A lesion tile, about 15 mm wide, cut from a 3D total-body photograph. A male subject, aged 53 to 57. The lesion is located on the upper back — 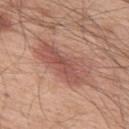Histopathological examination showed a dysplastic (Clark) nevus.